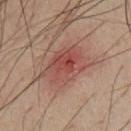workup: imaged on a skin check; not biopsied | illumination: cross-polarized illumination | TBP lesion metrics: an area of roughly 17 mm², a shape eccentricity near 0.75, and a symmetry-axis asymmetry near 0.2; a mean CIELAB color near L≈42 a*≈22 b*≈23 and a normalized lesion–skin contrast near 7; a color-variation rating of about 6.5/10 and radial color variation of about 2.5; an automated nevus-likeness rating near 15 out of 100 | subject: male, aged 48–52 | imaging modality: ~15 mm tile from a whole-body skin photo | location: the front of the torso | size: about 6 mm.